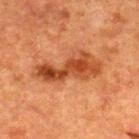| feature | finding |
|---|---|
| biopsy status | imaged on a skin check; not biopsied |
| illumination | cross-polarized illumination |
| TBP lesion metrics | a mean CIELAB color near L≈38 a*≈26 b*≈34, roughly 11 lightness units darker than nearby skin, and a normalized border contrast of about 9; a border-irregularity rating of about 5.5/10 and internal color variation of about 6 on a 0–10 scale; lesion-presence confidence of about 100/100 |
| image | ~15 mm crop, total-body skin-cancer survey |
| diameter | about 7.5 mm |
| subject | male, aged around 50 |
| anatomic site | the back |